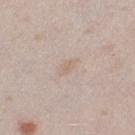| key | value |
|---|---|
| workup | catalogued during a skin exam; not biopsied |
| automated lesion analysis | a mean CIELAB color near L≈65 a*≈13 b*≈26, a lesion–skin lightness drop of about 5, and a lesion-to-skin contrast of about 4.5 (normalized; higher = more distinct); a within-lesion color-variation index near 1.5/10 and a peripheral color-asymmetry measure near 0.5 |
| imaging modality | 15 mm crop, total-body photography |
| body site | the right thigh |
| patient | female, about 25 years old |